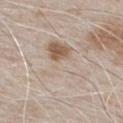The lesion was tiled from a total-body skin photograph and was not biopsied.
The lesion is located on the chest.
A roughly 15 mm field-of-view crop from a total-body skin photograph.
The lesion's longest dimension is about 7.5 mm.
A male subject in their 70s.